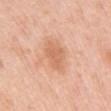Clinical summary:
A female subject in their 50s. Longest diameter approximately 4 mm. An algorithmic analysis of the crop reported an area of roughly 7 mm², a shape eccentricity near 0.75, and a symmetry-axis asymmetry near 0.25. The analysis additionally found a lesion color around L≈65 a*≈24 b*≈35 in CIELAB, about 9 CIELAB-L* units darker than the surrounding skin, and a normalized border contrast of about 5.5. It also reported a border-irregularity rating of about 2.5/10 and internal color variation of about 2.5 on a 0–10 scale. The analysis additionally found an automated nevus-likeness rating near 20 out of 100 and lesion-presence confidence of about 100/100. A 15 mm close-up extracted from a 3D total-body photography capture. The lesion is on the chest. The tile uses white-light illumination.